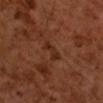biopsy status: total-body-photography surveillance lesion; no biopsy | site: the right upper arm | acquisition: ~15 mm crop, total-body skin-cancer survey | TBP lesion metrics: roughly 5 lightness units darker than nearby skin and a lesion-to-skin contrast of about 5.5 (normalized; higher = more distinct); border irregularity of about 3.5 on a 0–10 scale, a within-lesion color-variation index near 1.5/10, and peripheral color asymmetry of about 0.5; a nevus-likeness score of about 0/100 and a detector confidence of about 100 out of 100 that the crop contains a lesion | lesion size: ~3 mm (longest diameter) | lighting: cross-polarized | patient: male, aged approximately 60.